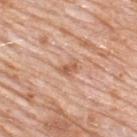Case summary:
• follow-up: catalogued during a skin exam; not biopsied
• TBP lesion metrics: an average lesion color of about L≈60 a*≈23 b*≈33 (CIELAB), about 10 CIELAB-L* units darker than the surrounding skin, and a lesion-to-skin contrast of about 6.5 (normalized; higher = more distinct); border irregularity of about 3.5 on a 0–10 scale, internal color variation of about 2.5 on a 0–10 scale, and a peripheral color-asymmetry measure near 1; an automated nevus-likeness rating near 0 out of 100 and a lesion-detection confidence of about 100/100
• patient: male, roughly 80 years of age
• site: the upper back
• lighting: white-light illumination
• image: ~15 mm tile from a whole-body skin photo